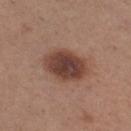<case>
<biopsy_status>not biopsied; imaged during a skin examination</biopsy_status>
<lesion_size>
  <long_diameter_mm_approx>5.0</long_diameter_mm_approx>
</lesion_size>
<image>
  <source>total-body photography crop</source>
  <field_of_view_mm>15</field_of_view_mm>
</image>
<automated_metrics>
  <area_mm2_approx>16.0</area_mm2_approx>
  <shape_asymmetry>0.15</shape_asymmetry>
  <cielab_L>42</cielab_L>
  <cielab_a>20</cielab_a>
  <cielab_b>25</cielab_b>
  <vs_skin_darker_L>14.0</vs_skin_darker_L>
  <vs_skin_contrast_norm>10.5</vs_skin_contrast_norm>
  <border_irregularity_0_10>1.5</border_irregularity_0_10>
  <color_variation_0_10>4.5</color_variation_0_10>
  <peripheral_color_asymmetry>1.0</peripheral_color_asymmetry>
</automated_metrics>
<patient>
  <sex>female</sex>
  <age_approx>30</age_approx>
</patient>
<lighting>white-light</lighting>
<site>right thigh</site>
</case>